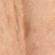Findings:
- follow-up · total-body-photography surveillance lesion; no biopsy
- illumination · cross-polarized illumination
- anatomic site · the mid back
- subject · female, aged 53 to 57
- acquisition · 15 mm crop, total-body photography